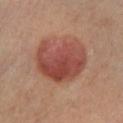follow-up — imaged on a skin check; not biopsied | anatomic site — the left lower leg | patient — male, aged around 70 | imaging modality — 15 mm crop, total-body photography.